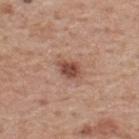Recorded during total-body skin imaging; not selected for excision or biopsy.
A male subject, approximately 60 years of age.
The lesion is on the upper back.
This is a white-light tile.
A 15 mm close-up extracted from a 3D total-body photography capture.